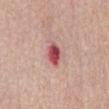biopsy status: imaged on a skin check; not biopsied | subject: female, aged around 65 | lesion diameter: about 3.5 mm | location: the abdomen | image: 15 mm crop, total-body photography | image-analysis metrics: a shape eccentricity near 0.7; lesion-presence confidence of about 100/100.